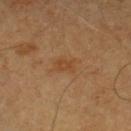Acquisition and patient details: This is a cross-polarized tile. A male patient aged around 60. Approximately 2.5 mm at its widest. The lesion is located on the chest. Automated tile analysis of the lesion measured a footprint of about 4 mm², an eccentricity of roughly 0.75, and a symmetry-axis asymmetry near 0.3. Cropped from a whole-body photographic skin survey; the tile spans about 15 mm.